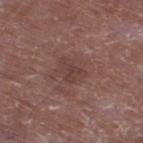Imaged during a routine full-body skin examination; the lesion was not biopsied and no histopathology is available.